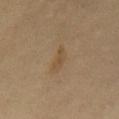{"biopsy_status": "not biopsied; imaged during a skin examination", "lighting": "cross-polarized", "site": "upper back", "image": {"source": "total-body photography crop", "field_of_view_mm": 15}, "automated_metrics": {"cielab_L": 45, "cielab_a": 13, "cielab_b": 30, "vs_skin_darker_L": 6.0, "vs_skin_contrast_norm": 5.5, "border_irregularity_0_10": 3.5, "color_variation_0_10": 1.5, "peripheral_color_asymmetry": 0.5, "nevus_likeness_0_100": 0, "lesion_detection_confidence_0_100": 100}, "lesion_size": {"long_diameter_mm_approx": 4.0}, "patient": {"sex": "male", "age_approx": 65}}